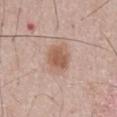Findings:
• workup: total-body-photography surveillance lesion; no biopsy
• tile lighting: white-light illumination
• size: about 3.5 mm
• imaging modality: ~15 mm crop, total-body skin-cancer survey
• subject: male, aged around 55
• image-analysis metrics: a border-irregularity index near 1.5/10 and a color-variation rating of about 3.5/10; a nevus-likeness score of about 95/100 and a lesion-detection confidence of about 100/100